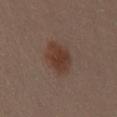{"biopsy_status": "not biopsied; imaged during a skin examination", "patient": {"sex": "female", "age_approx": 30}, "site": "mid back", "image": {"source": "total-body photography crop", "field_of_view_mm": 15}}